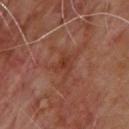Impression:
The lesion was photographed on a routine skin check and not biopsied; there is no pathology result.
Clinical summary:
Located on the upper back. Approximately 3 mm at its widest. The subject is a male aged 63 to 67. This is a cross-polarized tile. A 15 mm close-up extracted from a 3D total-body photography capture. Automated image analysis of the tile measured a mean CIELAB color near L≈36 a*≈24 b*≈29, about 6 CIELAB-L* units darker than the surrounding skin, and a lesion-to-skin contrast of about 6 (normalized; higher = more distinct). The analysis additionally found border irregularity of about 3.5 on a 0–10 scale, internal color variation of about 3 on a 0–10 scale, and radial color variation of about 1. The analysis additionally found an automated nevus-likeness rating near 0 out of 100 and lesion-presence confidence of about 100/100.